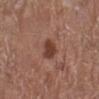follow-up: total-body-photography surveillance lesion; no biopsy | site: the left lower leg | acquisition: total-body-photography crop, ~15 mm field of view | patient: female, aged around 80.